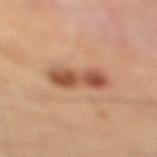notes: no biopsy performed (imaged during a skin exam); automated lesion analysis: a footprint of about 10 mm², an outline eccentricity of about 0.9 (0 = round, 1 = elongated), and two-axis asymmetry of about 0.2; acquisition: ~15 mm crop, total-body skin-cancer survey; illumination: cross-polarized; subject: male, aged approximately 40; anatomic site: the right lower leg.